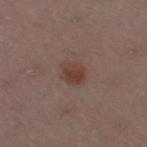Clinical impression:
The lesion was photographed on a routine skin check and not biopsied; there is no pathology result.
Image and clinical context:
The total-body-photography lesion software estimated a footprint of about 5.5 mm², a shape eccentricity near 0.65, and two-axis asymmetry of about 0.15. And it measured border irregularity of about 1.5 on a 0–10 scale, a within-lesion color-variation index near 3.5/10, and a peripheral color-asymmetry measure near 1. Imaged with white-light lighting. The recorded lesion diameter is about 3 mm. The patient is a female aged 53 to 57. A 15 mm close-up tile from a total-body photography series done for melanoma screening. Located on the right thigh.